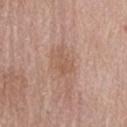Findings:
* workup: imaged on a skin check; not biopsied
* diameter: ~3.5 mm (longest diameter)
* anatomic site: the front of the torso
* subject: female, in their 70s
* imaging modality: total-body-photography crop, ~15 mm field of view
* illumination: white-light